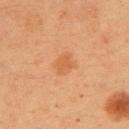Assessment: The lesion was photographed on a routine skin check and not biopsied; there is no pathology result. Background: The subject is a female roughly 40 years of age. About 3 mm across. Imaged with cross-polarized lighting. The lesion is on the arm. A 15 mm close-up tile from a total-body photography series done for melanoma screening.